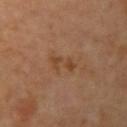Q: Was this lesion biopsied?
A: no biopsy performed (imaged during a skin exam)
Q: What is the imaging modality?
A: ~15 mm tile from a whole-body skin photo
Q: What is the lesion's diameter?
A: ≈3.5 mm
Q: How was the tile lit?
A: cross-polarized illumination
Q: Patient demographics?
A: female, aged around 60
Q: What is the anatomic site?
A: the left upper arm